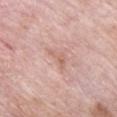| field | value |
|---|---|
| patient | male, about 75 years old |
| image-analysis metrics | a mean CIELAB color near L≈63 a*≈21 b*≈27, roughly 7 lightness units darker than nearby skin, and a normalized border contrast of about 5 |
| illumination | white-light |
| anatomic site | the chest |
| imaging modality | 15 mm crop, total-body photography |
| lesion size | ≈3 mm |